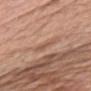This lesion was catalogued during total-body skin photography and was not selected for biopsy. Measured at roughly 3 mm in maximum diameter. Located on the chest. A female patient, approximately 65 years of age. A roughly 15 mm field-of-view crop from a total-body skin photograph. An algorithmic analysis of the crop reported a footprint of about 4 mm², an outline eccentricity of about 0.9 (0 = round, 1 = elongated), and a symmetry-axis asymmetry near 0.4. And it measured a mean CIELAB color near L≈56 a*≈20 b*≈30, a lesion–skin lightness drop of about 7, and a normalized lesion–skin contrast near 5. It also reported internal color variation of about 1 on a 0–10 scale and a peripheral color-asymmetry measure near 0. It also reported an automated nevus-likeness rating near 0 out of 100.